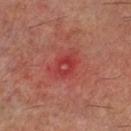Q: Was this lesion biopsied?
A: catalogued during a skin exam; not biopsied
Q: What lighting was used for the tile?
A: cross-polarized
Q: What is the imaging modality?
A: ~15 mm tile from a whole-body skin photo
Q: What are the patient's age and sex?
A: male, aged 58–62
Q: Lesion location?
A: the leg
Q: What did automated image analysis measure?
A: a footprint of about 4 mm² and an outline eccentricity of about 0.65 (0 = round, 1 = elongated); roughly 7 lightness units darker than nearby skin and a normalized lesion–skin contrast near 5.5
Q: How large is the lesion?
A: ~2.5 mm (longest diameter)